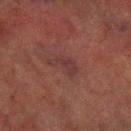{"biopsy_status": "not biopsied; imaged during a skin examination", "patient": {"sex": "male", "age_approx": 70}, "site": "left lower leg", "image": {"source": "total-body photography crop", "field_of_view_mm": 15}}